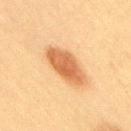Case summary:
* subject: female, about 50 years old
* automated lesion analysis: border irregularity of about 2 on a 0–10 scale, a color-variation rating of about 3.5/10, and a peripheral color-asymmetry measure near 1; a classifier nevus-likeness of about 100/100 and lesion-presence confidence of about 100/100
* location: the right thigh
* tile lighting: cross-polarized
* lesion size: ~5.5 mm (longest diameter)
* imaging modality: total-body-photography crop, ~15 mm field of view